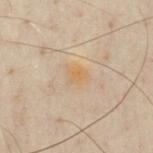Impression: This lesion was catalogued during total-body skin photography and was not selected for biopsy. Acquisition and patient details: About 3 mm across. A 15 mm crop from a total-body photograph taken for skin-cancer surveillance. The lesion is located on the chest. Imaged with cross-polarized lighting. A male subject aged approximately 50.